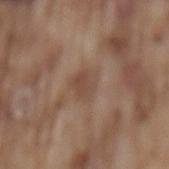Case summary:
– follow-up — imaged on a skin check; not biopsied
– automated metrics — a footprint of about 6.5 mm², an outline eccentricity of about 0.45 (0 = round, 1 = elongated), and a symmetry-axis asymmetry near 0.25; an average lesion color of about L≈47 a*≈17 b*≈27 (CIELAB), about 7 CIELAB-L* units darker than the surrounding skin, and a normalized lesion–skin contrast near 5.5
– image source — total-body-photography crop, ~15 mm field of view
– patient — male, approximately 75 years of age
– anatomic site — the back
– tile lighting — white-light illumination
– lesion size — ≈3 mm